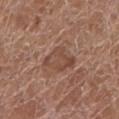The lesion was tiled from a total-body skin photograph and was not biopsied. Longest diameter approximately 4.5 mm. A female subject in their 80s. The lesion is on the left lower leg. Cropped from a whole-body photographic skin survey; the tile spans about 15 mm. Captured under white-light illumination.